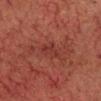follow-up = no biopsy performed (imaged during a skin exam) | patient = male, approximately 60 years of age | anatomic site = the head or neck | image = total-body-photography crop, ~15 mm field of view | TBP lesion metrics = an area of roughly 4 mm², a shape eccentricity near 0.65, and a symmetry-axis asymmetry near 0.45 | diameter = ~3 mm (longest diameter) | illumination = cross-polarized.